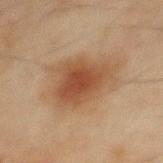biopsy status: no biopsy performed (imaged during a skin exam)
acquisition: ~15 mm crop, total-body skin-cancer survey
lighting: cross-polarized illumination
patient: male, aged 43 to 47
anatomic site: the mid back
automated lesion analysis: an average lesion color of about L≈41 a*≈17 b*≈28 (CIELAB) and a lesion-to-skin contrast of about 8 (normalized; higher = more distinct); internal color variation of about 4.5 on a 0–10 scale and a peripheral color-asymmetry measure near 1